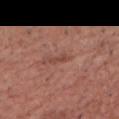follow-up = imaged on a skin check; not biopsied
lighting = white-light
image = 15 mm crop, total-body photography
subject = male, aged 63 to 67
diameter = about 2.5 mm
body site = the head or neck